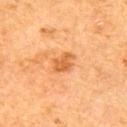Recorded during total-body skin imaging; not selected for excision or biopsy.
The lesion's longest dimension is about 3 mm.
The lesion is on the upper back.
A female subject aged around 60.
Imaged with cross-polarized lighting.
A 15 mm close-up extracted from a 3D total-body photography capture.